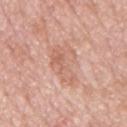<lesion>
<biopsy_status>not biopsied; imaged during a skin examination</biopsy_status>
<site>mid back</site>
<image>
  <source>total-body photography crop</source>
  <field_of_view_mm>15</field_of_view_mm>
</image>
<automated_metrics>
  <border_irregularity_0_10>4.5</border_irregularity_0_10>
  <color_variation_0_10>4.0</color_variation_0_10>
  <peripheral_color_asymmetry>1.5</peripheral_color_asymmetry>
</automated_metrics>
<lesion_size>
  <long_diameter_mm_approx>5.5</long_diameter_mm_approx>
</lesion_size>
<lighting>white-light</lighting>
<patient>
  <sex>male</sex>
  <age_approx>65</age_approx>
</patient>
</lesion>